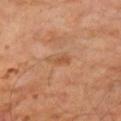Clinical impression:
Part of a total-body skin-imaging series; this lesion was reviewed on a skin check and was not flagged for biopsy.